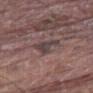| field | value |
|---|---|
| workup | imaged on a skin check; not biopsied |
| acquisition | ~15 mm tile from a whole-body skin photo |
| lesion diameter | ≈3 mm |
| location | the leg |
| patient | male, aged 78–82 |